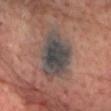This lesion was catalogued during total-body skin photography and was not selected for biopsy. A lesion tile, about 15 mm wide, cut from a 3D total-body photograph. The lesion is on the chest. Imaged with cross-polarized lighting. A male patient, roughly 65 years of age. About 7 mm across. Automated image analysis of the tile measured a footprint of about 23 mm², a shape eccentricity near 0.75, and a shape-asymmetry score of about 0.2 (0 = symmetric). The software also gave an average lesion color of about L≈41 a*≈8 b*≈14 (CIELAB), about 11 CIELAB-L* units darker than the surrounding skin, and a normalized lesion–skin contrast near 12.